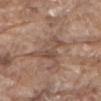{
  "lesion_size": {
    "long_diameter_mm_approx": 5.5
  },
  "patient": {
    "sex": "male",
    "age_approx": 80
  },
  "automated_metrics": {
    "cielab_L": 49,
    "cielab_a": 17,
    "cielab_b": 26,
    "vs_skin_darker_L": 8.0,
    "border_irregularity_0_10": 9.0,
    "peripheral_color_asymmetry": 1.0,
    "nevus_likeness_0_100": 0,
    "lesion_detection_confidence_0_100": 70
  },
  "lighting": "white-light",
  "site": "front of the torso",
  "image": {
    "source": "total-body photography crop",
    "field_of_view_mm": 15
  }
}